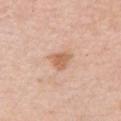Recorded during total-body skin imaging; not selected for excision or biopsy.
On the chest.
A 15 mm crop from a total-body photograph taken for skin-cancer surveillance.
The subject is a female aged 48 to 52.